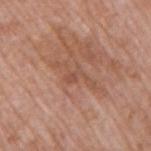Recorded during total-body skin imaging; not selected for excision or biopsy. The lesion is located on the right upper arm. A close-up tile cropped from a whole-body skin photograph, about 15 mm across. A male patient, aged 68–72. This is a white-light tile.